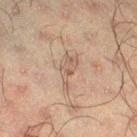This lesion was catalogued during total-body skin photography and was not selected for biopsy. About 5 mm across. This image is a 15 mm lesion crop taken from a total-body photograph. A male patient, in their 50s. Automated image analysis of the tile measured an average lesion color of about L≈45 a*≈13 b*≈22 (CIELAB), a lesion–skin lightness drop of about 6, and a lesion-to-skin contrast of about 5 (normalized; higher = more distinct). The analysis additionally found a border-irregularity rating of about 5/10, internal color variation of about 2.5 on a 0–10 scale, and a peripheral color-asymmetry measure near 1. The software also gave an automated nevus-likeness rating near 0 out of 100 and lesion-presence confidence of about 75/100. Captured under cross-polarized illumination. The lesion is on the right thigh.